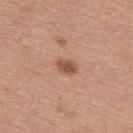Imaged during a routine full-body skin examination; the lesion was not biopsied and no histopathology is available. From the upper back. Automated image analysis of the tile measured two-axis asymmetry of about 0.25. And it measured a lesion color around L≈53 a*≈24 b*≈31 in CIELAB, about 11 CIELAB-L* units darker than the surrounding skin, and a normalized lesion–skin contrast near 8. The analysis additionally found a border-irregularity index near 2.5/10, a color-variation rating of about 3/10, and peripheral color asymmetry of about 1. A close-up tile cropped from a whole-body skin photograph, about 15 mm across. Imaged with white-light lighting. A male patient, in their mid- to late 50s. Approximately 2.5 mm at its widest.